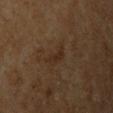Case summary:
– biopsy status · total-body-photography surveillance lesion; no biopsy
– automated metrics · a border-irregularity rating of about 4.5/10, a color-variation rating of about 0.5/10, and a peripheral color-asymmetry measure near 0; a classifier nevus-likeness of about 0/100 and lesion-presence confidence of about 100/100
– diameter · about 3 mm
– anatomic site · the right upper arm
– tile lighting · cross-polarized illumination
– acquisition · 15 mm crop, total-body photography
– subject · male, in their 60s